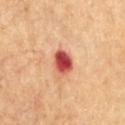This lesion was catalogued during total-body skin photography and was not selected for biopsy.
The patient is a male roughly 85 years of age or older.
Longest diameter approximately 3.5 mm.
This is a cross-polarized tile.
On the chest.
Cropped from a total-body skin-imaging series; the visible field is about 15 mm.